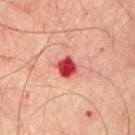Part of a total-body skin-imaging series; this lesion was reviewed on a skin check and was not flagged for biopsy. A lesion tile, about 15 mm wide, cut from a 3D total-body photograph. A male subject aged approximately 65. On the chest.